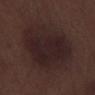Image and clinical context: An algorithmic analysis of the crop reported a lesion area of about 42 mm², a shape eccentricity near 0.7, and a symmetry-axis asymmetry near 0.2. A 15 mm crop from a total-body photograph taken for skin-cancer surveillance. The recorded lesion diameter is about 9 mm. The lesion is on the leg. The subject is a male approximately 70 years of age.